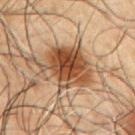Impression: The lesion was photographed on a routine skin check and not biopsied; there is no pathology result. Image and clinical context: This image is a 15 mm lesion crop taken from a total-body photograph. Located on the left upper arm. A male patient aged 48–52. Captured under cross-polarized illumination.